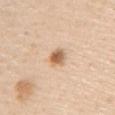Captured during whole-body skin photography for melanoma surveillance; the lesion was not biopsied. A lesion tile, about 15 mm wide, cut from a 3D total-body photograph. Imaged with white-light lighting. From the chest. The lesion's longest dimension is about 2.5 mm. A male patient, aged around 60.